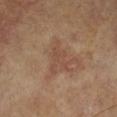| feature | finding |
|---|---|
| workup | total-body-photography surveillance lesion; no biopsy |
| tile lighting | cross-polarized |
| location | the leg |
| image source | ~15 mm crop, total-body skin-cancer survey |
| automated metrics | a footprint of about 6.5 mm², an outline eccentricity of about 0.85 (0 = round, 1 = elongated), and two-axis asymmetry of about 0.45; an automated nevus-likeness rating near 0 out of 100 and a detector confidence of about 100 out of 100 that the crop contains a lesion |
| patient | female, aged approximately 75 |